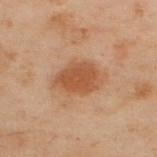Q: Was a biopsy performed?
A: total-body-photography surveillance lesion; no biopsy